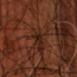  lesion_size:
    long_diameter_mm_approx: 2.5
  site: left forearm
  lighting: cross-polarized
  patient:
    sex: male
    age_approx: 70
  image:
    source: total-body photography crop
    field_of_view_mm: 15